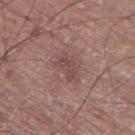This lesion was catalogued during total-body skin photography and was not selected for biopsy.
Cropped from a whole-body photographic skin survey; the tile spans about 15 mm.
The recorded lesion diameter is about 3 mm.
Imaged with white-light lighting.
Automated image analysis of the tile measured a lesion area of about 4 mm², an outline eccentricity of about 0.85 (0 = round, 1 = elongated), and a shape-asymmetry score of about 0.4 (0 = symmetric).
Located on the left thigh.
A male patient, about 75 years old.